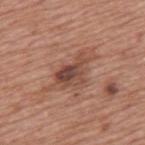Q: Automated lesion metrics?
A: a lesion area of about 14 mm², an eccentricity of roughly 0.85, and a symmetry-axis asymmetry near 0.45; a border-irregularity rating of about 6/10, a color-variation rating of about 7.5/10, and peripheral color asymmetry of about 2; a classifier nevus-likeness of about 50/100 and lesion-presence confidence of about 100/100
Q: What lighting was used for the tile?
A: white-light illumination
Q: What are the patient's age and sex?
A: male, in their mid-70s
Q: Where on the body is the lesion?
A: the back
Q: What is the lesion's diameter?
A: ~7 mm (longest diameter)
Q: What kind of image is this?
A: ~15 mm crop, total-body skin-cancer survey